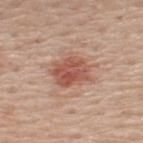Clinical summary:
Imaged with white-light lighting. A male patient, approximately 60 years of age. Automated image analysis of the tile measured an area of roughly 12 mm², an eccentricity of roughly 0.65, and a shape-asymmetry score of about 0.2 (0 = symmetric). The analysis additionally found a classifier nevus-likeness of about 90/100. A 15 mm close-up tile from a total-body photography series done for melanoma screening. The lesion is located on the upper back. Approximately 4.5 mm at its widest.